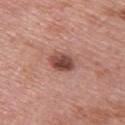Background:
A female subject, aged approximately 60. The lesion is on the upper back. A lesion tile, about 15 mm wide, cut from a 3D total-body photograph.